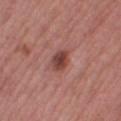No biopsy was performed on this lesion — it was imaged during a full skin examination and was not determined to be concerning. Approximately 3.5 mm at its widest. A region of skin cropped from a whole-body photographic capture, roughly 15 mm wide. The lesion is on the leg. The patient is a female aged 53 to 57. Captured under white-light illumination. The lesion-visualizer software estimated a lesion color around L≈43 a*≈25 b*≈24 in CIELAB, about 12 CIELAB-L* units darker than the surrounding skin, and a normalized lesion–skin contrast near 9. It also reported lesion-presence confidence of about 100/100.